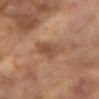Impression:
Recorded during total-body skin imaging; not selected for excision or biopsy.
Clinical summary:
An algorithmic analysis of the crop reported an average lesion color of about L≈43 a*≈19 b*≈29 (CIELAB), roughly 8 lightness units darker than nearby skin, and a normalized border contrast of about 6. It also reported border irregularity of about 4 on a 0–10 scale, internal color variation of about 3 on a 0–10 scale, and a peripheral color-asymmetry measure near 1. The software also gave a classifier nevus-likeness of about 0/100 and a detector confidence of about 100 out of 100 that the crop contains a lesion. The lesion is located on the right arm. Imaged with cross-polarized lighting. A region of skin cropped from a whole-body photographic capture, roughly 15 mm wide. A female patient, in their 80s.